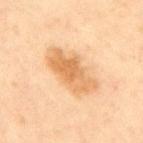notes = no biopsy performed (imaged during a skin exam)
location = the back
image = 15 mm crop, total-body photography
patient = male, aged around 45
diameter = ~7 mm (longest diameter)